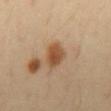workup: catalogued during a skin exam; not biopsied
image: 15 mm crop, total-body photography
tile lighting: cross-polarized
image-analysis metrics: a lesion area of about 8 mm², a shape eccentricity near 0.65, and a symmetry-axis asymmetry near 0.2; a border-irregularity rating of about 2/10, a color-variation rating of about 4/10, and peripheral color asymmetry of about 1
lesion size: about 3.5 mm
patient: male, aged around 40
location: the abdomen